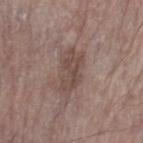The lesion was tiled from a total-body skin photograph and was not biopsied.
A region of skin cropped from a whole-body photographic capture, roughly 15 mm wide.
Imaged with white-light lighting.
A male patient, about 70 years old.
About 4.5 mm across.
From the left forearm.